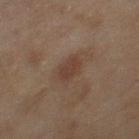biopsy status: total-body-photography surveillance lesion; no biopsy | body site: the right thigh | subject: female, aged 58–62 | acquisition: 15 mm crop, total-body photography.